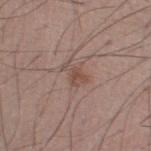workup=catalogued during a skin exam; not biopsied | subject=male, aged 53–57 | TBP lesion metrics=a mean CIELAB color near L≈49 a*≈18 b*≈24, roughly 8 lightness units darker than nearby skin, and a normalized lesion–skin contrast near 6; border irregularity of about 5.5 on a 0–10 scale, a color-variation rating of about 1/10, and a peripheral color-asymmetry measure near 0.5; an automated nevus-likeness rating near 20 out of 100 and lesion-presence confidence of about 100/100 | tile lighting=white-light | size=about 3 mm | imaging modality=~15 mm tile from a whole-body skin photo.